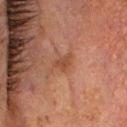Findings:
* workup: no biopsy performed (imaged during a skin exam)